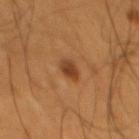Recorded during total-body skin imaging; not selected for excision or biopsy.
The tile uses cross-polarized illumination.
The lesion's longest dimension is about 2.5 mm.
A male patient roughly 60 years of age.
Located on the mid back.
A close-up tile cropped from a whole-body skin photograph, about 15 mm across.